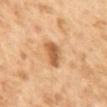Assessment: This lesion was catalogued during total-body skin photography and was not selected for biopsy. Clinical summary: Captured under cross-polarized illumination. On the upper back. The lesion-visualizer software estimated a detector confidence of about 100 out of 100 that the crop contains a lesion. Cropped from a total-body skin-imaging series; the visible field is about 15 mm. A female subject, aged approximately 60. About 4 mm across.